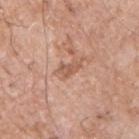Imaged during a routine full-body skin examination; the lesion was not biopsied and no histopathology is available. The tile uses white-light illumination. A 15 mm crop from a total-body photograph taken for skin-cancer surveillance. The lesion is on the chest. Longest diameter approximately 2.5 mm. The subject is a male aged around 75. The total-body-photography lesion software estimated an area of roughly 3 mm² and a shape eccentricity near 0.85. And it measured an average lesion color of about L≈57 a*≈22 b*≈30 (CIELAB), a lesion–skin lightness drop of about 9, and a lesion-to-skin contrast of about 6 (normalized; higher = more distinct). The software also gave a border-irregularity index near 4/10, a within-lesion color-variation index near 1/10, and peripheral color asymmetry of about 0.